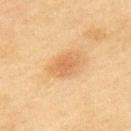Impression: The lesion was photographed on a routine skin check and not biopsied; there is no pathology result. Context: Captured under cross-polarized illumination. This image is a 15 mm lesion crop taken from a total-body photograph. The lesion is located on the upper back. The lesion's longest dimension is about 4 mm. The subject is a female aged around 60.